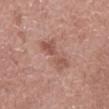{"biopsy_status": "not biopsied; imaged during a skin examination", "automated_metrics": {"cielab_L": 53, "cielab_a": 23, "cielab_b": 25, "vs_skin_darker_L": 9.0, "vs_skin_contrast_norm": 6.5, "border_irregularity_0_10": 6.0, "color_variation_0_10": 3.0, "peripheral_color_asymmetry": 1.0}, "site": "abdomen", "lighting": "white-light", "image": {"source": "total-body photography crop", "field_of_view_mm": 15}, "patient": {"sex": "male", "age_approx": 60}, "lesion_size": {"long_diameter_mm_approx": 4.5}}